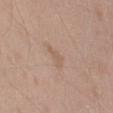Imaged during a routine full-body skin examination; the lesion was not biopsied and no histopathology is available. Located on the arm. A male subject in their mid- to late 40s. A 15 mm crop from a total-body photograph taken for skin-cancer surveillance. Longest diameter approximately 2.5 mm.